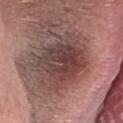This lesion was catalogued during total-body skin photography and was not selected for biopsy. A male patient, approximately 25 years of age. Longest diameter approximately 7.5 mm. The lesion is on the head or neck. A 15 mm close-up tile from a total-body photography series done for melanoma screening.